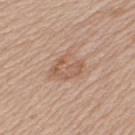Q: Is there a histopathology result?
A: total-body-photography surveillance lesion; no biopsy
Q: How was this image acquired?
A: total-body-photography crop, ~15 mm field of view
Q: What are the patient's age and sex?
A: female, in their mid-50s
Q: How large is the lesion?
A: about 4.5 mm
Q: What lighting was used for the tile?
A: white-light illumination
Q: Automated lesion metrics?
A: an average lesion color of about L≈58 a*≈19 b*≈29 (CIELAB), roughly 8 lightness units darker than nearby skin, and a normalized lesion–skin contrast near 5.5; border irregularity of about 3.5 on a 0–10 scale, internal color variation of about 3.5 on a 0–10 scale, and a peripheral color-asymmetry measure near 1
Q: What is the anatomic site?
A: the left upper arm